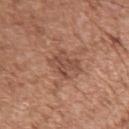Context:
The patient is a male aged 73–77. The recorded lesion diameter is about 4 mm. Located on the left upper arm. A region of skin cropped from a whole-body photographic capture, roughly 15 mm wide. The tile uses white-light illumination.